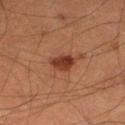This lesion was catalogued during total-body skin photography and was not selected for biopsy. A region of skin cropped from a whole-body photographic capture, roughly 15 mm wide. The patient is a male in their mid-60s. About 3 mm across. Automated image analysis of the tile measured a lesion-detection confidence of about 100/100. The lesion is on the left thigh. Captured under cross-polarized illumination.